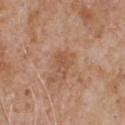follow-up: no biopsy performed (imaged during a skin exam)
automated metrics: an average lesion color of about L≈53 a*≈21 b*≈32 (CIELAB), roughly 7 lightness units darker than nearby skin, and a lesion-to-skin contrast of about 5 (normalized; higher = more distinct); a classifier nevus-likeness of about 0/100 and lesion-presence confidence of about 100/100
location: the chest
imaging modality: ~15 mm crop, total-body skin-cancer survey
illumination: white-light
patient: male, approximately 65 years of age